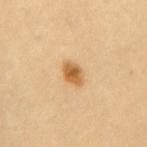Assessment: This lesion was catalogued during total-body skin photography and was not selected for biopsy. Context: A close-up tile cropped from a whole-body skin photograph, about 15 mm across. The recorded lesion diameter is about 2.5 mm. The lesion is located on the mid back. A female patient, aged 63 to 67. The tile uses cross-polarized illumination.